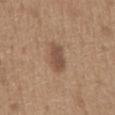The tile uses white-light illumination.
The subject is a male roughly 65 years of age.
Automated image analysis of the tile measured a lesion color around L≈50 a*≈17 b*≈28 in CIELAB and about 10 CIELAB-L* units darker than the surrounding skin. It also reported an automated nevus-likeness rating near 85 out of 100.
Approximately 4 mm at its widest.
From the front of the torso.
A 15 mm close-up tile from a total-body photography series done for melanoma screening.